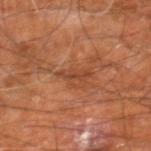Assessment: Recorded during total-body skin imaging; not selected for excision or biopsy. Background: The patient is a male about 60 years old. The lesion-visualizer software estimated a lesion area of about 3 mm², a shape eccentricity near 0.95, and two-axis asymmetry of about 0.5. The software also gave a lesion–skin lightness drop of about 8 and a lesion-to-skin contrast of about 6 (normalized; higher = more distinct). The software also gave a classifier nevus-likeness of about 0/100 and lesion-presence confidence of about 80/100. Cropped from a whole-body photographic skin survey; the tile spans about 15 mm. On the right leg.